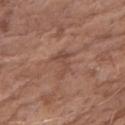follow-up: catalogued during a skin exam; not biopsied
subject: female, in their mid- to late 80s or older
image source: ~15 mm crop, total-body skin-cancer survey
anatomic site: the arm